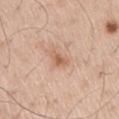Notes:
• workup · catalogued during a skin exam; not biopsied
• automated lesion analysis · a shape eccentricity near 0.9; a border-irregularity rating of about 3.5/10, a color-variation rating of about 1/10, and peripheral color asymmetry of about 0.5; an automated nevus-likeness rating near 10 out of 100 and lesion-presence confidence of about 100/100
• image · total-body-photography crop, ~15 mm field of view
• anatomic site · the leg
• illumination · white-light illumination
• lesion diameter · about 3 mm
• patient · male, about 60 years old